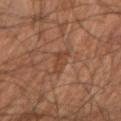biopsy status: no biopsy performed (imaged during a skin exam) | anatomic site: the right forearm | image: 15 mm crop, total-body photography | diameter: ~3 mm (longest diameter) | subject: male, aged approximately 60 | tile lighting: cross-polarized.